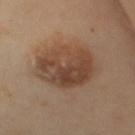biopsy_status: not biopsied; imaged during a skin examination
lesion_size:
  long_diameter_mm_approx: 7.0
image:
  source: total-body photography crop
  field_of_view_mm: 15
site: mid back
patient:
  sex: female
  age_approx: 55